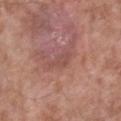The lesion was tiled from a total-body skin photograph and was not biopsied. A region of skin cropped from a whole-body photographic capture, roughly 15 mm wide. The subject is a male aged 53–57. Automated tile analysis of the lesion measured a lesion–skin lightness drop of about 6 and a normalized lesion–skin contrast near 4.5. The software also gave an automated nevus-likeness rating near 0 out of 100 and a lesion-detection confidence of about 95/100. From the right lower leg.